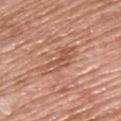  biopsy_status: not biopsied; imaged during a skin examination
  lighting: white-light
  patient:
    sex: male
    age_approx: 50
  lesion_size:
    long_diameter_mm_approx: 5.5
  image:
    source: total-body photography crop
    field_of_view_mm: 15
  automated_metrics:
    border_irregularity_0_10: 6.0
    color_variation_0_10: 4.5
  site: upper back